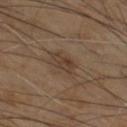The lesion was photographed on a routine skin check and not biopsied; there is no pathology result. Automated image analysis of the tile measured a footprint of about 5 mm², a shape eccentricity near 0.7, and two-axis asymmetry of about 0.3. And it measured a border-irregularity rating of about 3.5/10, internal color variation of about 3 on a 0–10 scale, and a peripheral color-asymmetry measure near 1. The lesion's longest dimension is about 3 mm. A close-up tile cropped from a whole-body skin photograph, about 15 mm across. Located on the right thigh. The tile uses cross-polarized illumination. A male subject, aged approximately 70.